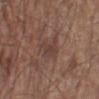follow-up: imaged on a skin check; not biopsied | size: ~3.5 mm (longest diameter) | patient: male, roughly 80 years of age | anatomic site: the left lower leg | imaging modality: ~15 mm tile from a whole-body skin photo | illumination: white-light illumination.